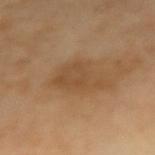Impression:
The lesion was photographed on a routine skin check and not biopsied; there is no pathology result.
Acquisition and patient details:
The lesion is located on the right forearm. A female subject aged 68 to 72. A region of skin cropped from a whole-body photographic capture, roughly 15 mm wide. Automated tile analysis of the lesion measured an average lesion color of about L≈49 a*≈19 b*≈35 (CIELAB) and about 7 CIELAB-L* units darker than the surrounding skin. It also reported border irregularity of about 4.5 on a 0–10 scale, internal color variation of about 2.5 on a 0–10 scale, and peripheral color asymmetry of about 1. It also reported a classifier nevus-likeness of about 0/100 and a lesion-detection confidence of about 100/100. The lesion's longest dimension is about 5 mm.